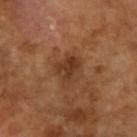• notes · catalogued during a skin exam; not biopsied
• site · the right forearm
• patient · female, about 70 years old
• lesion diameter · ~3 mm (longest diameter)
• imaging modality · total-body-photography crop, ~15 mm field of view